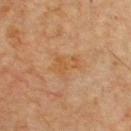Q: Was this lesion biopsied?
A: catalogued during a skin exam; not biopsied
Q: What are the patient's age and sex?
A: male, in their mid- to late 60s
Q: Where on the body is the lesion?
A: the chest
Q: What is the imaging modality?
A: 15 mm crop, total-body photography